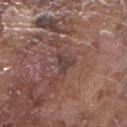This lesion was catalogued during total-body skin photography and was not selected for biopsy. Located on the right forearm. The lesion's longest dimension is about 2.5 mm. A male patient in their mid- to late 70s. Imaged with white-light lighting. A 15 mm close-up tile from a total-body photography series done for melanoma screening.